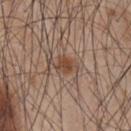The lesion was tiled from a total-body skin photograph and was not biopsied. The lesion-visualizer software estimated an area of roughly 4.5 mm², a shape eccentricity near 0.75, and a shape-asymmetry score of about 0.35 (0 = symmetric). The analysis additionally found a border-irregularity rating of about 3.5/10 and a color-variation rating of about 2.5/10. The lesion's longest dimension is about 3 mm. The patient is a male aged around 45. A 15 mm close-up tile from a total-body photography series done for melanoma screening. From the mid back. Captured under white-light illumination.